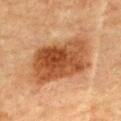Longest diameter approximately 8.5 mm.
Located on the back.
This is a cross-polarized tile.
A female subject in their 60s.
A region of skin cropped from a whole-body photographic capture, roughly 15 mm wide.